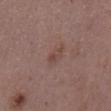The lesion was tiled from a total-body skin photograph and was not biopsied. Captured under white-light illumination. From the mid back. An algorithmic analysis of the crop reported an area of roughly 3.5 mm², a shape eccentricity near 0.85, and two-axis asymmetry of about 0.2. The software also gave an automated nevus-likeness rating near 0 out of 100. A 15 mm crop from a total-body photograph taken for skin-cancer surveillance. The patient is a male about 50 years old.